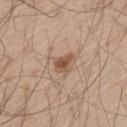Part of a total-body skin-imaging series; this lesion was reviewed on a skin check and was not flagged for biopsy.
This image is a 15 mm lesion crop taken from a total-body photograph.
A male subject, approximately 60 years of age.
On the left thigh.
Approximately 2.5 mm at its widest.
Captured under white-light illumination.